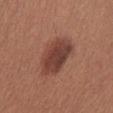Imaged during a routine full-body skin examination; the lesion was not biopsied and no histopathology is available.
The lesion is on the abdomen.
A 15 mm close-up extracted from a 3D total-body photography capture.
The tile uses white-light illumination.
A female subject approximately 25 years of age.
The lesion-visualizer software estimated an average lesion color of about L≈42 a*≈23 b*≈25 (CIELAB) and roughly 11 lightness units darker than nearby skin.
About 5.5 mm across.